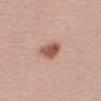Imaged during a routine full-body skin examination; the lesion was not biopsied and no histopathology is available.
From the left upper arm.
This image is a 15 mm lesion crop taken from a total-body photograph.
A female patient, in their mid- to late 40s.